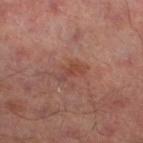Captured during whole-body skin photography for melanoma surveillance; the lesion was not biopsied. A roughly 15 mm field-of-view crop from a total-body skin photograph. The lesion is located on the left thigh. Captured under cross-polarized illumination. A male patient, aged approximately 65.